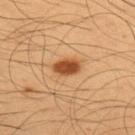  automated_metrics:
    border_irregularity_0_10: 2.0
    color_variation_0_10: 2.5
    peripheral_color_asymmetry: 1.0
    nevus_likeness_0_100: 100
    lesion_detection_confidence_0_100: 100
  lighting: cross-polarized
  patient:
    sex: male
    age_approx: 55
  site: upper back
  image:
    source: total-body photography crop
    field_of_view_mm: 15